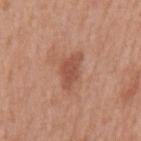<case>
<site>mid back</site>
<patient>
  <sex>male</sex>
  <age_approx>70</age_approx>
</patient>
<lighting>white-light</lighting>
<image>
  <source>total-body photography crop</source>
  <field_of_view_mm>15</field_of_view_mm>
</image>
</case>